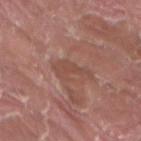Clinical impression:
Part of a total-body skin-imaging series; this lesion was reviewed on a skin check and was not flagged for biopsy.
Image and clinical context:
Automated image analysis of the tile measured a lesion–skin lightness drop of about 6 and a normalized border contrast of about 5. It also reported a border-irregularity index near 7.5/10, a within-lesion color-variation index near 1.5/10, and a peripheral color-asymmetry measure near 0.5. And it measured a classifier nevus-likeness of about 0/100 and a lesion-detection confidence of about 75/100. Cropped from a whole-body photographic skin survey; the tile spans about 15 mm. A male subject aged around 40. The lesion is on the right thigh.